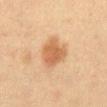workup = imaged on a skin check; not biopsied
lesion diameter = about 4 mm
patient = female, roughly 40 years of age
TBP lesion metrics = a normalized lesion–skin contrast near 7.5
image source = total-body-photography crop, ~15 mm field of view
anatomic site = the abdomen
lighting = cross-polarized illumination